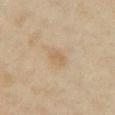This lesion was catalogued during total-body skin photography and was not selected for biopsy. A 15 mm close-up tile from a total-body photography series done for melanoma screening. The patient is a female aged 33–37. From the front of the torso.